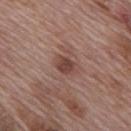Imaged during a routine full-body skin examination; the lesion was not biopsied and no histopathology is available. A male subject about 70 years old. Approximately 3 mm at its widest. Captured under white-light illumination. The lesion is on the mid back. Cropped from a total-body skin-imaging series; the visible field is about 15 mm.